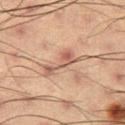Q: Is there a histopathology result?
A: imaged on a skin check; not biopsied
Q: Lesion location?
A: the right thigh
Q: What are the patient's age and sex?
A: male, about 55 years old
Q: How was the tile lit?
A: cross-polarized illumination
Q: What is the lesion's diameter?
A: ~5.5 mm (longest diameter)
Q: Automated lesion metrics?
A: a border-irregularity index near 7.5/10; an automated nevus-likeness rating near 5 out of 100
Q: What kind of image is this?
A: ~15 mm tile from a whole-body skin photo